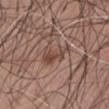A roughly 15 mm field-of-view crop from a total-body skin photograph.
This is a white-light tile.
A male subject, approximately 75 years of age.
From the abdomen.
The lesion-visualizer software estimated a mean CIELAB color near L≈45 a*≈19 b*≈24, about 9 CIELAB-L* units darker than the surrounding skin, and a normalized lesion–skin contrast near 6.5. The software also gave border irregularity of about 6 on a 0–10 scale, a within-lesion color-variation index near 3/10, and radial color variation of about 1. The software also gave a nevus-likeness score of about 25/100.
Longest diameter approximately 4.5 mm.